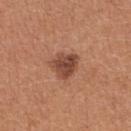follow-up: catalogued during a skin exam; not biopsied | patient: female, about 35 years old | acquisition: 15 mm crop, total-body photography | body site: the upper back.